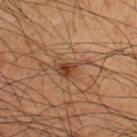Imaged with cross-polarized lighting. A roughly 15 mm field-of-view crop from a total-body skin photograph. The lesion's longest dimension is about 4 mm. The lesion-visualizer software estimated a footprint of about 7.5 mm². The analysis additionally found a nevus-likeness score of about 70/100. A male subject about 50 years old. Located on the upper back.